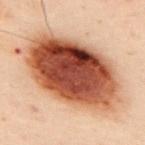biopsy_status: not biopsied; imaged during a skin examination
automated_metrics:
  nevus_likeness_0_100: 100
  lesion_detection_confidence_0_100: 100
lesion_size:
  long_diameter_mm_approx: 12.0
image:
  source: total-body photography crop
  field_of_view_mm: 15
site: upper back
lighting: cross-polarized
patient:
  sex: male
  age_approx: 50